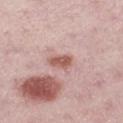Case summary:
- biopsy status — catalogued during a skin exam; not biopsied
- illumination — white-light illumination
- diameter — ~2.5 mm (longest diameter)
- patient — female, approximately 45 years of age
- TBP lesion metrics — an average lesion color of about L≈57 a*≈23 b*≈25 (CIELAB), a lesion–skin lightness drop of about 12, and a normalized lesion–skin contrast near 8.5; a border-irregularity rating of about 2.5/10 and radial color variation of about 1
- site — the leg
- image — ~15 mm tile from a whole-body skin photo